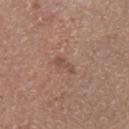image source: 15 mm crop, total-body photography; location: the leg; lesion size: ≈2.5 mm; tile lighting: white-light; patient: male, roughly 65 years of age.